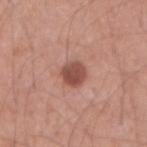Part of a total-body skin-imaging series; this lesion was reviewed on a skin check and was not flagged for biopsy.
About 3 mm across.
This is a white-light tile.
The lesion is located on the arm.
An algorithmic analysis of the crop reported an area of roughly 6 mm², an eccentricity of roughly 0.5, and a symmetry-axis asymmetry near 0.2. The software also gave a mean CIELAB color near L≈49 a*≈25 b*≈27 and about 13 CIELAB-L* units darker than the surrounding skin. The analysis additionally found a border-irregularity index near 1.5/10 and a peripheral color-asymmetry measure near 1.
A 15 mm close-up extracted from a 3D total-body photography capture.
A male subject roughly 35 years of age.